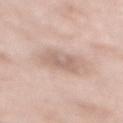The lesion was photographed on a routine skin check and not biopsied; there is no pathology result.
A female patient roughly 70 years of age.
On the mid back.
About 3.5 mm across.
Captured under white-light illumination.
A 15 mm close-up tile from a total-body photography series done for melanoma screening.
An algorithmic analysis of the crop reported an area of roughly 7.5 mm², an outline eccentricity of about 0.75 (0 = round, 1 = elongated), and a shape-asymmetry score of about 0.35 (0 = symmetric). The analysis additionally found a border-irregularity rating of about 4/10, a color-variation rating of about 1.5/10, and radial color variation of about 0.5.